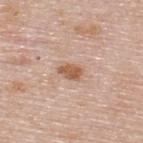{"biopsy_status": "not biopsied; imaged during a skin examination", "site": "upper back", "lesion_size": {"long_diameter_mm_approx": 2.5}, "lighting": "white-light", "image": {"source": "total-body photography crop", "field_of_view_mm": 15}, "patient": {"sex": "female", "age_approx": 50}}